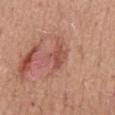Imaged during a routine full-body skin examination; the lesion was not biopsied and no histopathology is available.
This image is a 15 mm lesion crop taken from a total-body photograph.
Captured under white-light illumination.
Measured at roughly 2.5 mm in maximum diameter.
An algorithmic analysis of the crop reported a footprint of about 5 mm², a shape eccentricity near 0.65, and a symmetry-axis asymmetry near 0.35. It also reported internal color variation of about 2.5 on a 0–10 scale and a peripheral color-asymmetry measure near 1. And it measured a classifier nevus-likeness of about 5/100 and a detector confidence of about 100 out of 100 that the crop contains a lesion.
A male subject, roughly 50 years of age.
On the mid back.